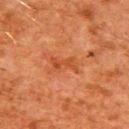workup — imaged on a skin check; not biopsied | subject — male, aged 78–82 | location — the right upper arm | tile lighting — cross-polarized illumination | acquisition — ~15 mm tile from a whole-body skin photo | lesion size — ≈3 mm | image-analysis metrics — a lesion area of about 5 mm², an eccentricity of roughly 0.85, and a shape-asymmetry score of about 0.3 (0 = symmetric).